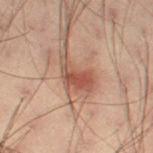Assessment: Part of a total-body skin-imaging series; this lesion was reviewed on a skin check and was not flagged for biopsy. Image and clinical context: Measured at roughly 4.5 mm in maximum diameter. A male subject roughly 55 years of age. This is a cross-polarized tile. A 15 mm crop from a total-body photograph taken for skin-cancer surveillance. The lesion is located on the leg.